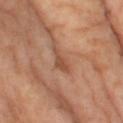workup=total-body-photography surveillance lesion; no biopsy
acquisition=total-body-photography crop, ~15 mm field of view
site=the left thigh
patient=female, roughly 70 years of age
lesion diameter=≈3 mm